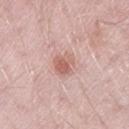workup = no biopsy performed (imaged during a skin exam) | acquisition = 15 mm crop, total-body photography | location = the left thigh | automated metrics = a border-irregularity index near 2.5/10 and internal color variation of about 4 on a 0–10 scale | subject = male, aged approximately 50 | diameter = about 3 mm.